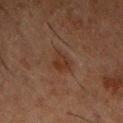Clinical impression:
The lesion was photographed on a routine skin check and not biopsied; there is no pathology result.
Image and clinical context:
On the chest. Longest diameter approximately 3 mm. A male subject aged approximately 50. A 15 mm crop from a total-body photograph taken for skin-cancer surveillance.